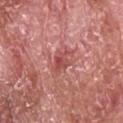Impression: The lesion was tiled from a total-body skin photograph and was not biopsied. Clinical summary: The lesion's longest dimension is about 3.5 mm. An algorithmic analysis of the crop reported a mean CIELAB color near L≈51 a*≈31 b*≈25, about 9 CIELAB-L* units darker than the surrounding skin, and a lesion-to-skin contrast of about 6.5 (normalized; higher = more distinct). It also reported a border-irregularity rating of about 4.5/10, a color-variation rating of about 4/10, and peripheral color asymmetry of about 1. The tile uses white-light illumination. The subject is a male approximately 65 years of age. Cropped from a total-body skin-imaging series; the visible field is about 15 mm. The lesion is located on the back.